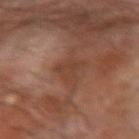Impression: Recorded during total-body skin imaging; not selected for excision or biopsy. Clinical summary: This image is a 15 mm lesion crop taken from a total-body photograph. The subject is a male aged approximately 65. From the right forearm.